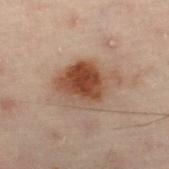follow-up — no biopsy performed (imaged during a skin exam)
tile lighting — cross-polarized
image source — total-body-photography crop, ~15 mm field of view
patient — male, approximately 50 years of age
automated lesion analysis — a shape eccentricity near 0.65 and a shape-asymmetry score of about 0.2 (0 = symmetric); a mean CIELAB color near L≈39 a*≈17 b*≈25, about 11 CIELAB-L* units darker than the surrounding skin, and a normalized border contrast of about 9.5; a border-irregularity rating of about 2.5/10, a within-lesion color-variation index near 7/10, and radial color variation of about 2
body site — the left leg